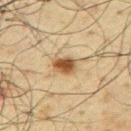<lesion>
  <biopsy_status>not biopsied; imaged during a skin examination</biopsy_status>
  <automated_metrics>
    <eccentricity>0.75</eccentricity>
    <shape_asymmetry>0.25</shape_asymmetry>
    <cielab_L>42</cielab_L>
    <cielab_a>14</cielab_a>
    <cielab_b>30</cielab_b>
    <vs_skin_contrast_norm>10.5</vs_skin_contrast_norm>
    <border_irregularity_0_10>2.0</border_irregularity_0_10>
    <color_variation_0_10>4.5</color_variation_0_10>
    <peripheral_color_asymmetry>1.5</peripheral_color_asymmetry>
    <nevus_likeness_0_100>95</nevus_likeness_0_100>
    <lesion_detection_confidence_0_100>100</lesion_detection_confidence_0_100>
  </automated_metrics>
  <lighting>cross-polarized</lighting>
  <site>front of the torso</site>
  <patient>
    <sex>male</sex>
    <age_approx>60</age_approx>
  </patient>
  <image>
    <source>total-body photography crop</source>
    <field_of_view_mm>15</field_of_view_mm>
  </image>
</lesion>